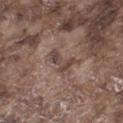Q: Is there a histopathology result?
A: no biopsy performed (imaged during a skin exam)
Q: What are the patient's age and sex?
A: male, aged approximately 75
Q: What is the anatomic site?
A: the left thigh
Q: What did automated image analysis measure?
A: a lesion area of about 4.5 mm², an outline eccentricity of about 0.85 (0 = round, 1 = elongated), and two-axis asymmetry of about 0.6; a border-irregularity rating of about 6.5/10, a color-variation rating of about 3.5/10, and radial color variation of about 1
Q: Lesion size?
A: ≈3.5 mm
Q: How was this image acquired?
A: ~15 mm crop, total-body skin-cancer survey
Q: Illumination type?
A: white-light illumination